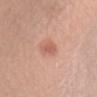biopsy_status: not biopsied; imaged during a skin examination